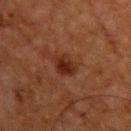Captured during whole-body skin photography for melanoma surveillance; the lesion was not biopsied. A male subject aged around 50. The lesion's longest dimension is about 3 mm. This is a cross-polarized tile. An algorithmic analysis of the crop reported a lesion area of about 5 mm² and a shape-asymmetry score of about 0.25 (0 = symmetric). The software also gave an average lesion color of about L≈22 a*≈20 b*≈24 (CIELAB) and a normalized border contrast of about 9. The analysis additionally found a border-irregularity rating of about 2/10 and a peripheral color-asymmetry measure near 1. The lesion is located on the upper back. A region of skin cropped from a whole-body photographic capture, roughly 15 mm wide.